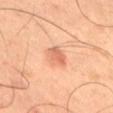This lesion was catalogued during total-body skin photography and was not selected for biopsy. A male subject, aged approximately 50. A lesion tile, about 15 mm wide, cut from a 3D total-body photograph. The lesion's longest dimension is about 2.5 mm. The lesion is located on the right thigh.